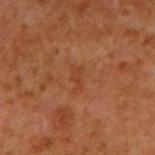Recorded during total-body skin imaging; not selected for excision or biopsy.